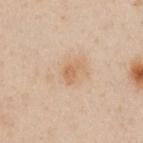* biopsy status: imaged on a skin check; not biopsied
* subject: male, roughly 50 years of age
* body site: the left arm
* lighting: cross-polarized
* acquisition: ~15 mm crop, total-body skin-cancer survey